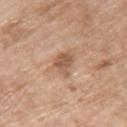Captured during whole-body skin photography for melanoma surveillance; the lesion was not biopsied.